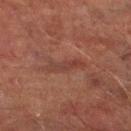Clinical impression:
The lesion was photographed on a routine skin check and not biopsied; there is no pathology result.
Clinical summary:
The lesion is located on the right lower leg. Imaged with cross-polarized lighting. A roughly 15 mm field-of-view crop from a total-body skin photograph. A male subject aged 73 to 77. The lesion-visualizer software estimated a normalized lesion–skin contrast near 5. And it measured a border-irregularity index near 5/10, a within-lesion color-variation index near 3/10, and peripheral color asymmetry of about 1. And it measured a lesion-detection confidence of about 55/100. The lesion's longest dimension is about 4.5 mm.